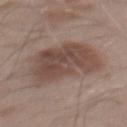Captured during whole-body skin photography for melanoma surveillance; the lesion was not biopsied. Automated tile analysis of the lesion measured an average lesion color of about L≈47 a*≈16 b*≈23 (CIELAB), about 11 CIELAB-L* units darker than the surrounding skin, and a normalized lesion–skin contrast near 8.5. And it measured a border-irregularity rating of about 3.5/10 and peripheral color asymmetry of about 1.5. The lesion's longest dimension is about 8 mm. This image is a 15 mm lesion crop taken from a total-body photograph. On the mid back. A male patient, approximately 55 years of age. Imaged with white-light lighting.